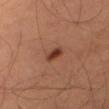Imaged during a routine full-body skin examination; the lesion was not biopsied and no histopathology is available.
Located on the left thigh.
The lesion's longest dimension is about 2.5 mm.
A male patient, in their mid- to late 60s.
A 15 mm close-up tile from a total-body photography series done for melanoma screening.
The total-body-photography lesion software estimated about 11 CIELAB-L* units darker than the surrounding skin and a lesion-to-skin contrast of about 9.5 (normalized; higher = more distinct). And it measured a border-irregularity index near 1.5/10, internal color variation of about 2.5 on a 0–10 scale, and radial color variation of about 1.
The tile uses cross-polarized illumination.